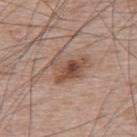Imaged during a routine full-body skin examination; the lesion was not biopsied and no histopathology is available.
The patient is a male roughly 65 years of age.
From the upper back.
A 15 mm crop from a total-body photograph taken for skin-cancer surveillance.